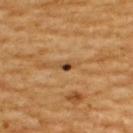{"biopsy_status": "not biopsied; imaged during a skin examination", "patient": {"sex": "male", "age_approx": 60}, "image": {"source": "total-body photography crop", "field_of_view_mm": 15}, "lesion_size": {"long_diameter_mm_approx": 1.5}, "automated_metrics": {"eccentricity": 0.8, "shape_asymmetry": 0.2, "cielab_L": 39, "cielab_a": 20, "cielab_b": 34, "vs_skin_darker_L": 16.0, "vs_skin_contrast_norm": 13.0, "border_irregularity_0_10": 1.5, "nevus_likeness_0_100": 85, "lesion_detection_confidence_0_100": 100}, "site": "upper back", "lighting": "cross-polarized"}